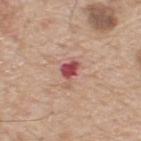{"biopsy_status": "not biopsied; imaged during a skin examination", "patient": {"sex": "male", "age_approx": 70}, "image": {"source": "total-body photography crop", "field_of_view_mm": 15}, "lighting": "white-light", "automated_metrics": {"border_irregularity_0_10": 5.0, "color_variation_0_10": 2.5}, "lesion_size": {"long_diameter_mm_approx": 3.0}, "site": "upper back"}